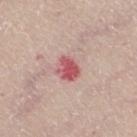Impression: Imaged during a routine full-body skin examination; the lesion was not biopsied and no histopathology is available. Image and clinical context: Imaged with white-light lighting. An algorithmic analysis of the crop reported an outline eccentricity of about 0.4 (0 = round, 1 = elongated) and a symmetry-axis asymmetry near 0.25. And it measured a lesion color around L≈55 a*≈32 b*≈22 in CIELAB, roughly 13 lightness units darker than nearby skin, and a normalized border contrast of about 9. Cropped from a total-body skin-imaging series; the visible field is about 15 mm. The lesion's longest dimension is about 3 mm. A female patient, approximately 45 years of age. On the right thigh.